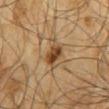- illumination · cross-polarized
- subject · male, about 65 years old
- automated lesion analysis · a lesion area of about 7.5 mm², an eccentricity of roughly 0.8, and a shape-asymmetry score of about 0.25 (0 = symmetric); a lesion color around L≈44 a*≈17 b*≈34 in CIELAB, a lesion–skin lightness drop of about 12, and a normalized border contrast of about 9
- anatomic site · the mid back
- diameter · ≈4 mm
- image · total-body-photography crop, ~15 mm field of view